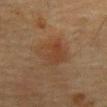follow-up=total-body-photography surveillance lesion; no biopsy.